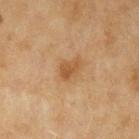This lesion was catalogued during total-body skin photography and was not selected for biopsy.
An algorithmic analysis of the crop reported a shape-asymmetry score of about 0.35 (0 = symmetric). The software also gave a mean CIELAB color near L≈48 a*≈20 b*≈37, about 8 CIELAB-L* units darker than the surrounding skin, and a normalized border contrast of about 7. It also reported a border-irregularity index near 3/10, internal color variation of about 1.5 on a 0–10 scale, and a peripheral color-asymmetry measure near 0.5.
Imaged with cross-polarized lighting.
The lesion is located on the left upper arm.
A 15 mm crop from a total-body photograph taken for skin-cancer surveillance.
Approximately 2.5 mm at its widest.
The patient is a female about 60 years old.